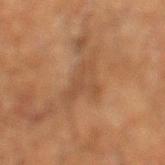Clinical summary:
Cropped from a total-body skin-imaging series; the visible field is about 15 mm. On the left lower leg. The recorded lesion diameter is about 4 mm. A male subject about 65 years old.